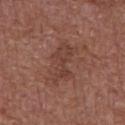Case summary:
• notes · imaged on a skin check; not biopsied